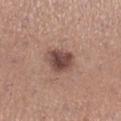notes: total-body-photography surveillance lesion; no biopsy
body site: the right lower leg
size: ≈4 mm
acquisition: total-body-photography crop, ~15 mm field of view
patient: female, aged around 30